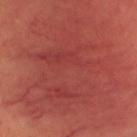Q: Lesion location?
A: the head or neck
Q: Lesion size?
A: ≈13 mm
Q: What did automated image analysis measure?
A: a lesion color around L≈42 a*≈33 b*≈27 in CIELAB, roughly 6 lightness units darker than nearby skin, and a normalized border contrast of about 5.5; border irregularity of about 7.5 on a 0–10 scale and peripheral color asymmetry of about 1.5; an automated nevus-likeness rating near 0 out of 100
Q: What kind of image is this?
A: total-body-photography crop, ~15 mm field of view
Q: Who is the patient?
A: male, in their mid- to late 30s
Q: Illumination type?
A: cross-polarized